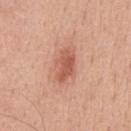Q: Is there a histopathology result?
A: no biopsy performed (imaged during a skin exam)
Q: What is the imaging modality?
A: 15 mm crop, total-body photography
Q: Lesion location?
A: the chest
Q: What are the patient's age and sex?
A: male, roughly 50 years of age
Q: Automated lesion metrics?
A: an outline eccentricity of about 0.85 (0 = round, 1 = elongated) and a shape-asymmetry score of about 0.25 (0 = symmetric); an average lesion color of about L≈57 a*≈27 b*≈31 (CIELAB) and a lesion-to-skin contrast of about 7 (normalized; higher = more distinct); a border-irregularity rating of about 2.5/10 and a within-lesion color-variation index near 3.5/10; a detector confidence of about 100 out of 100 that the crop contains a lesion